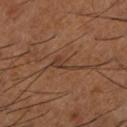{"biopsy_status": "not biopsied; imaged during a skin examination", "image": {"source": "total-body photography crop", "field_of_view_mm": 15}, "patient": {"sex": "male", "age_approx": 65}, "automated_metrics": {"area_mm2_approx": 4.5, "shape_asymmetry": 0.55, "vs_skin_contrast_norm": 6.0, "border_irregularity_0_10": 5.5, "peripheral_color_asymmetry": 0.5}, "site": "left lower leg", "lighting": "cross-polarized"}